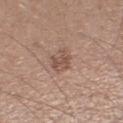A 15 mm close-up tile from a total-body photography series done for melanoma screening.
A male patient aged 63 to 67.
The lesion is on the right lower leg.
About 3 mm across.